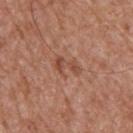<tbp_lesion>
  <biopsy_status>not biopsied; imaged during a skin examination</biopsy_status>
  <image>
    <source>total-body photography crop</source>
    <field_of_view_mm>15</field_of_view_mm>
  </image>
  <patient>
    <sex>male</sex>
    <age_approx>65</age_approx>
  </patient>
  <site>upper back</site>
  <lighting>white-light</lighting>
</tbp_lesion>